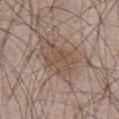Q: Was a biopsy performed?
A: imaged on a skin check; not biopsied
Q: How was this image acquired?
A: total-body-photography crop, ~15 mm field of view
Q: Lesion location?
A: the front of the torso
Q: Patient demographics?
A: male, roughly 65 years of age